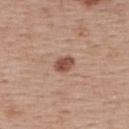Located on the upper back. A female patient, in their mid-50s. Automated tile analysis of the lesion measured a border-irregularity index near 2.5/10 and internal color variation of about 2.5 on a 0–10 scale. And it measured a lesion-detection confidence of about 100/100. This is a white-light tile. Cropped from a total-body skin-imaging series; the visible field is about 15 mm.